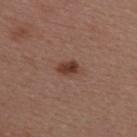Assessment: The lesion was tiled from a total-body skin photograph and was not biopsied. Image and clinical context: The lesion-visualizer software estimated an automated nevus-likeness rating near 95 out of 100 and a lesion-detection confidence of about 100/100. The lesion is on the right upper arm. A female subject approximately 45 years of age. The tile uses white-light illumination. The recorded lesion diameter is about 3 mm. A lesion tile, about 15 mm wide, cut from a 3D total-body photograph.